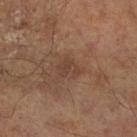The lesion was photographed on a routine skin check and not biopsied; there is no pathology result.
The patient is a male aged approximately 65.
On the right lower leg.
A 15 mm close-up extracted from a 3D total-body photography capture.